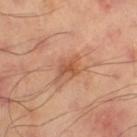Assessment:
This lesion was catalogued during total-body skin photography and was not selected for biopsy.
Clinical summary:
About 3 mm across. Imaged with cross-polarized lighting. A 15 mm close-up extracted from a 3D total-body photography capture. The lesion is on the left thigh. The total-body-photography lesion software estimated a lesion area of about 4.5 mm², a shape eccentricity near 0.7, and a symmetry-axis asymmetry near 0.4. It also reported a nevus-likeness score of about 30/100 and a lesion-detection confidence of about 100/100.